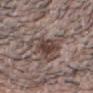Q: How was this image acquired?
A: ~15 mm tile from a whole-body skin photo
Q: What are the patient's age and sex?
A: male, aged 33 to 37
Q: How was the tile lit?
A: white-light illumination
Q: Lesion location?
A: the head or neck